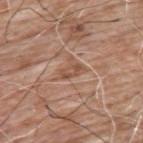Assessment:
Imaged during a routine full-body skin examination; the lesion was not biopsied and no histopathology is available.
Image and clinical context:
From the upper back. The subject is a male aged around 60. The lesion's longest dimension is about 2.5 mm. Imaged with white-light lighting. A 15 mm crop from a total-body photograph taken for skin-cancer surveillance.